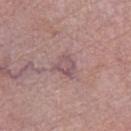The lesion was tiled from a total-body skin photograph and was not biopsied.
A 15 mm crop from a total-body photograph taken for skin-cancer surveillance.
From the right thigh.
Imaged with white-light lighting.
The patient is a female in their 70s.
The lesion's longest dimension is about 2.5 mm.